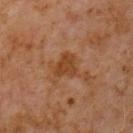<lesion>
  <biopsy_status>not biopsied; imaged during a skin examination</biopsy_status>
  <patient>
    <sex>male</sex>
    <age_approx>60</age_approx>
  </patient>
  <site>chest</site>
  <image>
    <source>total-body photography crop</source>
    <field_of_view_mm>15</field_of_view_mm>
  </image>
</lesion>